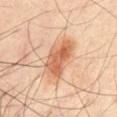Q: Was this lesion biopsied?
A: catalogued during a skin exam; not biopsied
Q: What is the anatomic site?
A: the abdomen
Q: What kind of image is this?
A: 15 mm crop, total-body photography
Q: What did automated image analysis measure?
A: a footprint of about 12 mm²
Q: Lesion size?
A: ~5.5 mm (longest diameter)
Q: Patient demographics?
A: male, about 45 years old